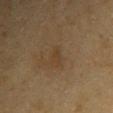Q: Was a biopsy performed?
A: imaged on a skin check; not biopsied
Q: What is the imaging modality?
A: ~15 mm tile from a whole-body skin photo
Q: Lesion location?
A: the right upper arm
Q: Patient demographics?
A: female, approximately 55 years of age